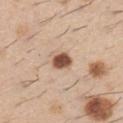The lesion was photographed on a routine skin check and not biopsied; there is no pathology result.
This is a white-light tile.
A male patient aged 58–62.
A close-up tile cropped from a whole-body skin photograph, about 15 mm across.
The lesion is on the right upper arm.
Longest diameter approximately 2.5 mm.